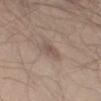follow-up: no biopsy performed (imaged during a skin exam) | imaging modality: 15 mm crop, total-body photography | diameter: ~3 mm (longest diameter) | patient: male, roughly 45 years of age | site: the arm.